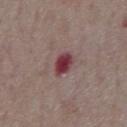The lesion was tiled from a total-body skin photograph and was not biopsied.
This image is a 15 mm lesion crop taken from a total-body photograph.
The total-body-photography lesion software estimated an outline eccentricity of about 0.85 (0 = round, 1 = elongated) and a symmetry-axis asymmetry near 0.2. And it measured a lesion color around L≈38 a*≈28 b*≈17 in CIELAB, roughly 15 lightness units darker than nearby skin, and a lesion-to-skin contrast of about 12 (normalized; higher = more distinct). The software also gave a color-variation rating of about 3.5/10 and a peripheral color-asymmetry measure near 1.
The lesion's longest dimension is about 3.5 mm.
The subject is a male aged around 75.
The lesion is on the chest.
The tile uses white-light illumination.